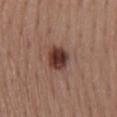anatomic site: the mid back; imaging modality: ~15 mm tile from a whole-body skin photo; patient: female, aged 53 to 57; tile lighting: white-light; size: ≈3.5 mm.